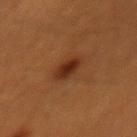Clinical impression:
This lesion was catalogued during total-body skin photography and was not selected for biopsy.
Context:
The recorded lesion diameter is about 3.5 mm. A male subject, roughly 60 years of age. The lesion is on the lower back. The total-body-photography lesion software estimated an area of roughly 6 mm² and an outline eccentricity of about 0.8 (0 = round, 1 = elongated). And it measured about 9 CIELAB-L* units darker than the surrounding skin. The software also gave a nevus-likeness score of about 100/100 and a detector confidence of about 100 out of 100 that the crop contains a lesion. Imaged with cross-polarized lighting. A 15 mm crop from a total-body photograph taken for skin-cancer surveillance.